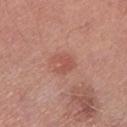This lesion was catalogued during total-body skin photography and was not selected for biopsy. Located on the left lower leg. The lesion's longest dimension is about 3 mm. The subject is a male aged 48–52. A roughly 15 mm field-of-view crop from a total-body skin photograph.